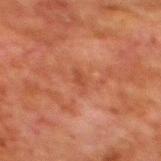<case>
<biopsy_status>not biopsied; imaged during a skin examination</biopsy_status>
<automated_metrics>
  <area_mm2_approx>3.0</area_mm2_approx>
  <shape_asymmetry>0.35</shape_asymmetry>
  <cielab_L>38</cielab_L>
  <cielab_a>24</cielab_a>
  <cielab_b>29</cielab_b>
  <vs_skin_contrast_norm>4.5</vs_skin_contrast_norm>
  <border_irregularity_0_10>3.5</border_irregularity_0_10>
  <color_variation_0_10>1.0</color_variation_0_10>
  <nevus_likeness_0_100>0</nevus_likeness_0_100>
  <lesion_detection_confidence_0_100>100</lesion_detection_confidence_0_100>
</automated_metrics>
<image>
  <source>total-body photography crop</source>
  <field_of_view_mm>15</field_of_view_mm>
</image>
<patient>
  <sex>male</sex>
  <age_approx>80</age_approx>
</patient>
<site>mid back</site>
<lesion_size>
  <long_diameter_mm_approx>2.5</long_diameter_mm_approx>
</lesion_size>
<lighting>cross-polarized</lighting>
</case>